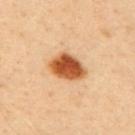biopsy status — imaged on a skin check; not biopsied | site — the upper back | illumination — cross-polarized illumination | imaging modality — 15 mm crop, total-body photography | lesion diameter — ≈4.5 mm | patient — male, approximately 40 years of age.